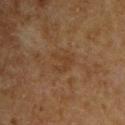notes — total-body-photography surveillance lesion; no biopsy | anatomic site — the upper back | image source — ~15 mm crop, total-body skin-cancer survey | TBP lesion metrics — a lesion area of about 3.5 mm², an outline eccentricity of about 0.45 (0 = round, 1 = elongated), and a symmetry-axis asymmetry near 0.5; a detector confidence of about 100 out of 100 that the crop contains a lesion | patient — male, about 65 years old.